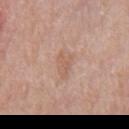  site: mid back
  image:
    source: total-body photography crop
    field_of_view_mm: 15
  lighting: white-light
  patient:
    sex: male
    age_approx: 70